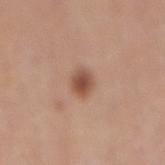notes=no biopsy performed (imaged during a skin exam); anatomic site=the lower back; image source=15 mm crop, total-body photography; lighting=white-light illumination; diameter=about 2.5 mm; subject=male, approximately 70 years of age.